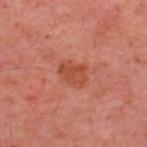workup: imaged on a skin check; not biopsied
subject: approximately 55 years of age
site: the upper back
image: 15 mm crop, total-body photography